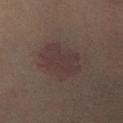| feature | finding |
|---|---|
| workup | imaged on a skin check; not biopsied |
| lesion size | ~5 mm (longest diameter) |
| subject | female, aged approximately 60 |
| image | total-body-photography crop, ~15 mm field of view |
| anatomic site | the left forearm |
| image-analysis metrics | a lesion area of about 16 mm², a shape eccentricity near 0.5, and a symmetry-axis asymmetry near 0.2; a mean CIELAB color near L≈31 a*≈13 b*≈14, a lesion–skin lightness drop of about 5, and a lesion-to-skin contrast of about 6 (normalized; higher = more distinct); a border-irregularity rating of about 2.5/10 and peripheral color asymmetry of about 0.5; a classifier nevus-likeness of about 30/100 and a lesion-detection confidence of about 100/100 |